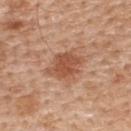Located on the upper back. A 15 mm crop from a total-body photograph taken for skin-cancer surveillance. A male patient, aged 58–62.